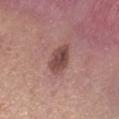follow-up: no biopsy performed (imaged during a skin exam) | tile lighting: white-light illumination | image source: 15 mm crop, total-body photography | lesion size: ~4 mm (longest diameter) | image-analysis metrics: an eccentricity of roughly 0.8 and a shape-asymmetry score of about 0.2 (0 = symmetric); an average lesion color of about L≈47 a*≈22 b*≈22 (CIELAB), roughly 12 lightness units darker than nearby skin, and a normalized lesion–skin contrast near 8.5; internal color variation of about 4.5 on a 0–10 scale and radial color variation of about 1.5; a nevus-likeness score of about 50/100 and lesion-presence confidence of about 100/100 | patient: female, in their mid-30s | body site: the right forearm.